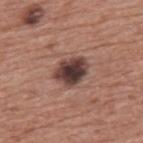{
  "biopsy_status": "not biopsied; imaged during a skin examination",
  "automated_metrics": {
    "cielab_L": 39,
    "cielab_a": 19,
    "cielab_b": 20,
    "vs_skin_darker_L": 17.0,
    "vs_skin_contrast_norm": 14.0,
    "border_irregularity_0_10": 2.0,
    "color_variation_0_10": 6.0,
    "nevus_likeness_0_100": 15,
    "lesion_detection_confidence_0_100": 100
  },
  "patient": {
    "sex": "male",
    "age_approx": 75
  },
  "lesion_size": {
    "long_diameter_mm_approx": 4.0
  },
  "lighting": "white-light",
  "site": "upper back",
  "image": {
    "source": "total-body photography crop",
    "field_of_view_mm": 15
  }
}